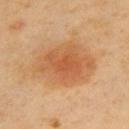Part of a total-body skin-imaging series; this lesion was reviewed on a skin check and was not flagged for biopsy. A female patient in their 50s. The recorded lesion diameter is about 7.5 mm. Automated tile analysis of the lesion measured a lesion color around L≈57 a*≈23 b*≈39 in CIELAB. On the upper back. A 15 mm crop from a total-body photograph taken for skin-cancer surveillance.